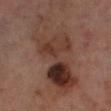Captured during whole-body skin photography for melanoma surveillance; the lesion was not biopsied. This image is a 15 mm lesion crop taken from a total-body photograph. Captured under cross-polarized illumination. A male subject, aged around 70. The lesion-visualizer software estimated an area of roughly 38 mm², an eccentricity of roughly 0.9, and a shape-asymmetry score of about 0.4 (0 = symmetric). The analysis additionally found a lesion color around L≈35 a*≈19 b*≈24 in CIELAB. It also reported a border-irregularity rating of about 7/10. Located on the left lower leg.